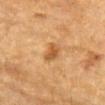The lesion was photographed on a routine skin check and not biopsied; there is no pathology result. A 15 mm crop from a total-body photograph taken for skin-cancer surveillance. A male subject, roughly 85 years of age or older. The lesion is on the left forearm. Approximately 2.5 mm at its widest. Imaged with cross-polarized lighting.